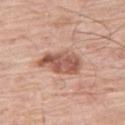Assessment: No biopsy was performed on this lesion — it was imaged during a full skin examination and was not determined to be concerning. Context: Longest diameter approximately 5.5 mm. An algorithmic analysis of the crop reported a lesion color around L≈56 a*≈23 b*≈28 in CIELAB and a lesion-to-skin contrast of about 8.5 (normalized; higher = more distinct). It also reported an automated nevus-likeness rating near 45 out of 100 and lesion-presence confidence of about 100/100. A male subject approximately 80 years of age. The lesion is located on the leg. Captured under white-light illumination. A 15 mm close-up extracted from a 3D total-body photography capture.